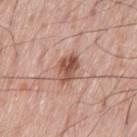On the left thigh. The subject is a male aged 58 to 62. A 15 mm close-up tile from a total-body photography series done for melanoma screening.